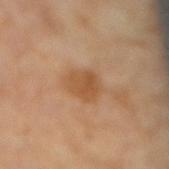| feature | finding |
|---|---|
| biopsy status | no biopsy performed (imaged during a skin exam) |
| patient | male, aged around 70 |
| imaging modality | 15 mm crop, total-body photography |
| diameter | ~3 mm (longest diameter) |
| tile lighting | cross-polarized |
| body site | the lower back |